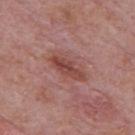workup = catalogued during a skin exam; not biopsied
site = the mid back
subject = male, about 75 years old
lighting = white-light illumination
imaging modality = ~15 mm tile from a whole-body skin photo